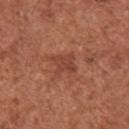This lesion was catalogued during total-body skin photography and was not selected for biopsy. The lesion is on the right upper arm. Automated tile analysis of the lesion measured a lesion color around L≈43 a*≈27 b*≈30 in CIELAB, roughly 7 lightness units darker than nearby skin, and a normalized lesion–skin contrast near 6. It also reported a border-irregularity index near 3.5/10, internal color variation of about 2 on a 0–10 scale, and radial color variation of about 0.5. The lesion's longest dimension is about 3 mm. Captured under white-light illumination. A 15 mm close-up extracted from a 3D total-body photography capture. A female patient about 50 years old.